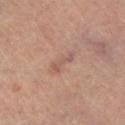This lesion was catalogued during total-body skin photography and was not selected for biopsy.
This is a cross-polarized tile.
A male subject, in their 60s.
A 15 mm close-up extracted from a 3D total-body photography capture.
Approximately 3.5 mm at its widest.
From the right lower leg.